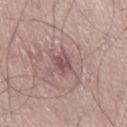A male subject, in their mid-50s. A 15 mm crop from a total-body photograph taken for skin-cancer surveillance. The lesion is located on the right thigh. Longest diameter approximately 2.5 mm. This is a white-light tile. Automated tile analysis of the lesion measured an area of roughly 4 mm² and two-axis asymmetry of about 0.25.